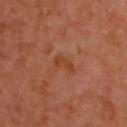notes=total-body-photography surveillance lesion; no biopsy | patient=female, aged around 40 | lesion size=about 3 mm | image=15 mm crop, total-body photography | automated metrics=a lesion area of about 3 mm² and two-axis asymmetry of about 0.4; a nevus-likeness score of about 0/100 | location=the back | tile lighting=cross-polarized illumination.